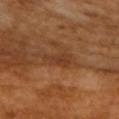Clinical impression:
No biopsy was performed on this lesion — it was imaged during a full skin examination and was not determined to be concerning.
Clinical summary:
Approximately 3.5 mm at its widest. A 15 mm close-up tile from a total-body photography series done for melanoma screening. The total-body-photography lesion software estimated border irregularity of about 6 on a 0–10 scale and a within-lesion color-variation index near 1/10. Captured under cross-polarized illumination. The patient is a male in their mid-60s.